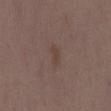Captured during whole-body skin photography for melanoma surveillance; the lesion was not biopsied. Automated tile analysis of the lesion measured about 5 CIELAB-L* units darker than the surrounding skin and a normalized border contrast of about 6. The software also gave internal color variation of about 0 on a 0–10 scale and radial color variation of about 0. The analysis additionally found an automated nevus-likeness rating near 0 out of 100 and a lesion-detection confidence of about 100/100. Located on the lower back. Captured under white-light illumination. A female subject, aged 33–37. Longest diameter approximately 2.5 mm. A lesion tile, about 15 mm wide, cut from a 3D total-body photograph.